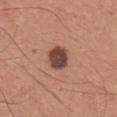notes=no biopsy performed (imaged during a skin exam); subject=male, approximately 35 years of age; image source=~15 mm crop, total-body skin-cancer survey; lesion diameter=about 3 mm; site=the arm.